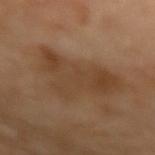biopsy status: catalogued during a skin exam; not biopsied
lesion size: about 7.5 mm
image source: ~15 mm crop, total-body skin-cancer survey
subject: female, approximately 70 years of age
location: the left forearm
image-analysis metrics: a lesion area of about 25 mm² and a symmetry-axis asymmetry near 0.55; a mean CIELAB color near L≈42 a*≈17 b*≈32 and a lesion-to-skin contrast of about 6.5 (normalized; higher = more distinct)